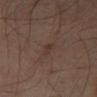Cropped from a whole-body photographic skin survey; the tile spans about 15 mm. The patient is a male aged 63–67. From the left thigh. Automated image analysis of the tile measured an average lesion color of about L≈32 a*≈15 b*≈22 (CIELAB), a lesion–skin lightness drop of about 5, and a lesion-to-skin contrast of about 6 (normalized; higher = more distinct). The software also gave border irregularity of about 4 on a 0–10 scale, internal color variation of about 0 on a 0–10 scale, and radial color variation of about 0. It also reported a classifier nevus-likeness of about 0/100 and a lesion-detection confidence of about 100/100. The tile uses cross-polarized illumination.